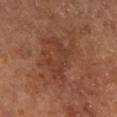site: the right lower leg | automated metrics: a mean CIELAB color near L≈38 a*≈24 b*≈29; a border-irregularity rating of about 9/10, a within-lesion color-variation index near 1.5/10, and peripheral color asymmetry of about 0.5; a nevus-likeness score of about 5/100 and lesion-presence confidence of about 100/100 | patient: male, roughly 65 years of age | acquisition: ~15 mm crop, total-body skin-cancer survey | illumination: cross-polarized.